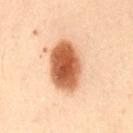Captured during whole-body skin photography for melanoma surveillance; the lesion was not biopsied.
Cropped from a whole-body photographic skin survey; the tile spans about 15 mm.
The subject is a male aged approximately 55.
Longest diameter approximately 6 mm.
Captured under cross-polarized illumination.
The lesion is located on the back.